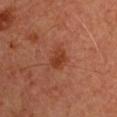biopsy_status: not biopsied; imaged during a skin examination
patient:
  sex: male
  age_approx: 65
lesion_size:
  long_diameter_mm_approx: 2.5
lighting: cross-polarized
image:
  source: total-body photography crop
  field_of_view_mm: 15
automated_metrics:
  cielab_L: 34
  cielab_a: 26
  cielab_b: 31
  vs_skin_darker_L: 8.0
  vs_skin_contrast_norm: 7.5
  border_irregularity_0_10: 2.5
  peripheral_color_asymmetry: 0.5
  nevus_likeness_0_100: 50
  lesion_detection_confidence_0_100: 100
site: right upper arm